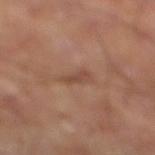Notes:
* follow-up: imaged on a skin check; not biopsied
* TBP lesion metrics: a footprint of about 3 mm², an outline eccentricity of about 0.9 (0 = round, 1 = elongated), and a symmetry-axis asymmetry near 0.35
* illumination: cross-polarized illumination
* site: the right thigh
* image: ~15 mm crop, total-body skin-cancer survey
* lesion size: ≈3 mm